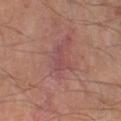Findings:
• notes — catalogued during a skin exam; not biopsied